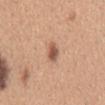This lesion was catalogued during total-body skin photography and was not selected for biopsy. A female patient, approximately 30 years of age. Captured under white-light illumination. A 15 mm close-up tile from a total-body photography series done for melanoma screening. On the mid back. Automated tile analysis of the lesion measured a lesion color around L≈55 a*≈22 b*≈31 in CIELAB, roughly 13 lightness units darker than nearby skin, and a lesion-to-skin contrast of about 8.5 (normalized; higher = more distinct). The software also gave border irregularity of about 2.5 on a 0–10 scale, a color-variation rating of about 2.5/10, and peripheral color asymmetry of about 1. The lesion's longest dimension is about 3 mm.